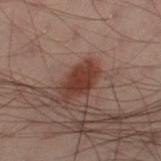follow-up — no biopsy performed (imaged during a skin exam)
illumination — cross-polarized
patient — male, about 40 years old
imaging modality — ~15 mm tile from a whole-body skin photo
site — the left thigh